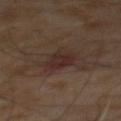{"biopsy_status": "not biopsied; imaged during a skin examination", "lighting": "cross-polarized", "patient": {"sex": "male", "age_approx": 65}, "image": {"source": "total-body photography crop", "field_of_view_mm": 15}, "automated_metrics": {"cielab_L": 26, "cielab_a": 16, "cielab_b": 18, "vs_skin_contrast_norm": 7.0, "border_irregularity_0_10": 2.5, "color_variation_0_10": 4.0, "peripheral_color_asymmetry": 1.5}}